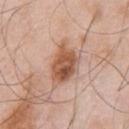Impression:
Captured during whole-body skin photography for melanoma surveillance; the lesion was not biopsied.
Background:
Longest diameter approximately 5 mm. The patient is a male aged approximately 55. On the chest. This image is a 15 mm lesion crop taken from a total-body photograph. The total-body-photography lesion software estimated a footprint of about 12 mm², an outline eccentricity of about 0.75 (0 = round, 1 = elongated), and two-axis asymmetry of about 0.25. And it measured a mean CIELAB color near L≈55 a*≈23 b*≈32 and about 14 CIELAB-L* units darker than the surrounding skin. It also reported a classifier nevus-likeness of about 85/100 and a lesion-detection confidence of about 100/100.